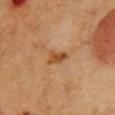Q: Is there a histopathology result?
A: total-body-photography surveillance lesion; no biopsy
Q: Illumination type?
A: cross-polarized
Q: What is the anatomic site?
A: the chest
Q: Automated lesion metrics?
A: an eccentricity of roughly 0.8 and two-axis asymmetry of about 0.25; a mean CIELAB color near L≈40 a*≈20 b*≈33 and roughly 9 lightness units darker than nearby skin; an automated nevus-likeness rating near 30 out of 100 and lesion-presence confidence of about 100/100
Q: Who is the patient?
A: female, aged 48 to 52
Q: What kind of image is this?
A: 15 mm crop, total-body photography
Q: Lesion size?
A: about 3 mm